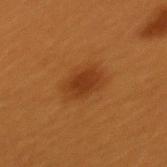| feature | finding |
|---|---|
| patient | female, aged 28 to 32 |
| size | ≈4 mm |
| site | the upper back |
| image | total-body-photography crop, ~15 mm field of view |
| tile lighting | cross-polarized |
| automated lesion analysis | a lesion color around L≈35 a*≈24 b*≈37 in CIELAB and a lesion–skin lightness drop of about 8; a border-irregularity index near 2/10 and peripheral color asymmetry of about 0.5 |